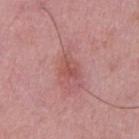Findings:
* biopsy status · catalogued during a skin exam; not biopsied
* patient · male, about 50 years old
* image-analysis metrics · a border-irregularity index near 3/10 and internal color variation of about 2.5 on a 0–10 scale
* imaging modality · ~15 mm tile from a whole-body skin photo
* location · the left upper arm